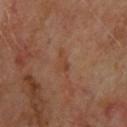{
  "biopsy_status": "not biopsied; imaged during a skin examination",
  "patient": {
    "sex": "male",
    "age_approx": 65
  },
  "lesion_size": {
    "long_diameter_mm_approx": 2.5
  },
  "site": "upper back",
  "lighting": "cross-polarized",
  "image": {
    "source": "total-body photography crop",
    "field_of_view_mm": 15
  }
}